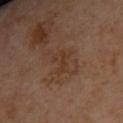biopsy status: total-body-photography surveillance lesion; no biopsy | lighting: cross-polarized illumination | automated lesion analysis: a lesion area of about 4.5 mm², an eccentricity of roughly 0.8, and a shape-asymmetry score of about 0.4 (0 = symmetric); a nevus-likeness score of about 0/100 | image source: ~15 mm tile from a whole-body skin photo | site: the back | subject: female, aged 38 to 42.